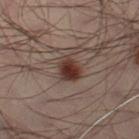Recorded during total-body skin imaging; not selected for excision or biopsy. This is a cross-polarized tile. A lesion tile, about 15 mm wide, cut from a 3D total-body photograph. The recorded lesion diameter is about 3.5 mm. A male patient, aged around 40. The lesion is located on the leg.